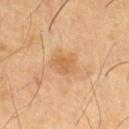Assessment: Captured during whole-body skin photography for melanoma surveillance; the lesion was not biopsied. Image and clinical context: The lesion is on the leg. Cropped from a total-body skin-imaging series; the visible field is about 15 mm. An algorithmic analysis of the crop reported an area of roughly 5.5 mm² and a shape eccentricity near 0.7. The software also gave an average lesion color of about L≈62 a*≈22 b*≈40 (CIELAB), a lesion–skin lightness drop of about 8, and a lesion-to-skin contrast of about 6 (normalized; higher = more distinct). The software also gave border irregularity of about 2 on a 0–10 scale and a color-variation rating of about 2/10. The patient is a male aged approximately 65. The tile uses cross-polarized illumination.